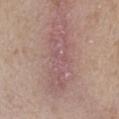The lesion was photographed on a routine skin check and not biopsied; there is no pathology result.
A 15 mm crop from a total-body photograph taken for skin-cancer surveillance.
A female patient about 60 years old.
The lesion's longest dimension is about 6.5 mm.
The lesion is located on the left forearm.
An algorithmic analysis of the crop reported a border-irregularity index near 8/10. The software also gave a classifier nevus-likeness of about 0/100 and a detector confidence of about 60 out of 100 that the crop contains a lesion.